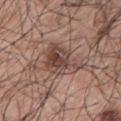{
  "biopsy_status": "not biopsied; imaged during a skin examination",
  "patient": {
    "sex": "male",
    "age_approx": 70
  },
  "lesion_size": {
    "long_diameter_mm_approx": 5.5
  },
  "site": "arm",
  "image": {
    "source": "total-body photography crop",
    "field_of_view_mm": 15
  }
}